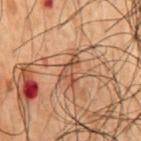Assessment:
Recorded during total-body skin imaging; not selected for excision or biopsy.
Image and clinical context:
The lesion is located on the mid back. A roughly 15 mm field-of-view crop from a total-body skin photograph. A male subject, roughly 50 years of age. The recorded lesion diameter is about 3.5 mm.